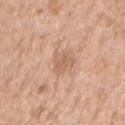subject = female, aged 83 or over; diameter = ~3 mm (longest diameter); TBP lesion metrics = a border-irregularity rating of about 3/10, a color-variation rating of about 2/10, and peripheral color asymmetry of about 0.5; imaging modality = ~15 mm crop, total-body skin-cancer survey; site = the right upper arm.